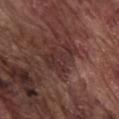Findings:
– follow-up — no biopsy performed (imaged during a skin exam)
– diameter — about 4.5 mm
– image-analysis metrics — a border-irregularity index near 6.5/10, internal color variation of about 2 on a 0–10 scale, and a peripheral color-asymmetry measure near 1
– patient — male, approximately 75 years of age
– tile lighting — white-light
– anatomic site — the front of the torso
– image source — 15 mm crop, total-body photography